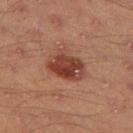{
  "lesion_size": {
    "long_diameter_mm_approx": 4.5
  },
  "patient": {
    "sex": "male",
    "age_approx": 45
  },
  "image": {
    "source": "total-body photography crop",
    "field_of_view_mm": 15
  },
  "site": "left thigh",
  "lighting": "cross-polarized"
}